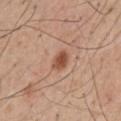Background:
A male patient, aged approximately 55. A region of skin cropped from a whole-body photographic capture, roughly 15 mm wide. From the mid back. The lesion's longest dimension is about 2.5 mm.